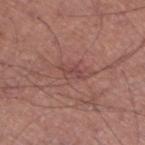workup: no biopsy performed (imaged during a skin exam) | subject: male, approximately 45 years of age | lesion size: about 2.5 mm | tile lighting: white-light | image: 15 mm crop, total-body photography | anatomic site: the right lower leg | image-analysis metrics: a border-irregularity rating of about 5.5/10, internal color variation of about 1.5 on a 0–10 scale, and a peripheral color-asymmetry measure near 0.5; a classifier nevus-likeness of about 0/100 and a lesion-detection confidence of about 95/100.